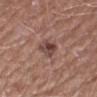The lesion was photographed on a routine skin check and not biopsied; there is no pathology result.
About 3.5 mm across.
A male patient, in their mid-70s.
Captured under white-light illumination.
A 15 mm close-up extracted from a 3D total-body photography capture.
On the right forearm.